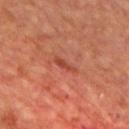Recorded during total-body skin imaging; not selected for excision or biopsy. A 15 mm close-up tile from a total-body photography series done for melanoma screening. On the back. Approximately 3 mm at its widest. A male patient, in their mid- to late 60s. Automated tile analysis of the lesion measured a footprint of about 2.5 mm² and a symmetry-axis asymmetry near 0.4. The analysis additionally found border irregularity of about 4.5 on a 0–10 scale. The analysis additionally found a classifier nevus-likeness of about 0/100 and a lesion-detection confidence of about 95/100.